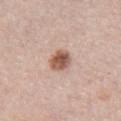<tbp_lesion>
<biopsy_status>not biopsied; imaged during a skin examination</biopsy_status>
<image>
  <source>total-body photography crop</source>
  <field_of_view_mm>15</field_of_view_mm>
</image>
<site>front of the torso</site>
<patient>
  <sex>female</sex>
  <age_approx>65</age_approx>
</patient>
<automated_metrics>
  <area_mm2_approx>5.5</area_mm2_approx>
  <eccentricity>0.45</eccentricity>
  <shape_asymmetry>0.2</shape_asymmetry>
  <nevus_likeness_0_100>100</nevus_likeness_0_100>
</automated_metrics>
<lighting>white-light</lighting>
</tbp_lesion>